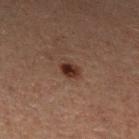site: the leg
imaging modality: ~15 mm crop, total-body skin-cancer survey
TBP lesion metrics: a lesion area of about 4.5 mm², a shape eccentricity near 0.65, and a symmetry-axis asymmetry near 0.25; a lesion color around L≈24 a*≈15 b*≈19 in CIELAB, a lesion–skin lightness drop of about 9, and a lesion-to-skin contrast of about 9.5 (normalized; higher = more distinct); a detector confidence of about 100 out of 100 that the crop contains a lesion
patient: male, roughly 60 years of age
illumination: cross-polarized illumination
size: about 2.5 mm